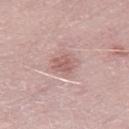This lesion was catalogued during total-body skin photography and was not selected for biopsy.
A male subject, about 50 years old.
A lesion tile, about 15 mm wide, cut from a 3D total-body photograph.
The recorded lesion diameter is about 2.5 mm.
From the right thigh.